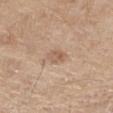Clinical impression: This lesion was catalogued during total-body skin photography and was not selected for biopsy. Clinical summary: Captured under white-light illumination. Cropped from a total-body skin-imaging series; the visible field is about 15 mm. The patient is a male about 70 years old. From the right thigh.